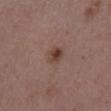Captured during whole-body skin photography for melanoma surveillance; the lesion was not biopsied.
This is a white-light tile.
A male subject in their 50s.
The lesion is located on the mid back.
The lesion's longest dimension is about 2.5 mm.
A region of skin cropped from a whole-body photographic capture, roughly 15 mm wide.